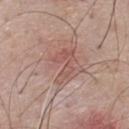Assessment:
The lesion was photographed on a routine skin check and not biopsied; there is no pathology result.
Image and clinical context:
An algorithmic analysis of the crop reported an area of roughly 6.5 mm², an eccentricity of roughly 0.85, and two-axis asymmetry of about 0.55. It also reported an average lesion color of about L≈54 a*≈22 b*≈24 (CIELAB) and a normalized border contrast of about 5.5. Imaged with white-light lighting. A male patient about 45 years old. The lesion is located on the upper back. A close-up tile cropped from a whole-body skin photograph, about 15 mm across.